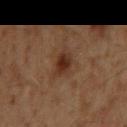biopsy_status: not biopsied; imaged during a skin examination
patient:
  sex: male
  age_approx: 60
image:
  source: total-body photography crop
  field_of_view_mm: 15
site: mid back